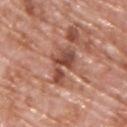Q: Is there a histopathology result?
A: catalogued during a skin exam; not biopsied
Q: What is the lesion's diameter?
A: ~4 mm (longest diameter)
Q: Patient demographics?
A: male, roughly 70 years of age
Q: What lighting was used for the tile?
A: white-light
Q: Lesion location?
A: the upper back
Q: How was this image acquired?
A: total-body-photography crop, ~15 mm field of view